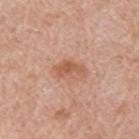workup: catalogued during a skin exam; not biopsied | size: about 3.5 mm | automated metrics: a lesion color around L≈57 a*≈24 b*≈33 in CIELAB and about 10 CIELAB-L* units darker than the surrounding skin; border irregularity of about 5 on a 0–10 scale; a classifier nevus-likeness of about 35/100 and a lesion-detection confidence of about 100/100 | anatomic site: the arm | illumination: white-light illumination | subject: male, aged 78 to 82 | image: 15 mm crop, total-body photography.